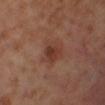Recorded during total-body skin imaging; not selected for excision or biopsy. The recorded lesion diameter is about 3.5 mm. Imaged with cross-polarized lighting. An algorithmic analysis of the crop reported a mean CIELAB color near L≈36 a*≈21 b*≈26 and a lesion–skin lightness drop of about 7. It also reported a border-irregularity rating of about 2.5/10 and radial color variation of about 1. And it measured a detector confidence of about 100 out of 100 that the crop contains a lesion. A lesion tile, about 15 mm wide, cut from a 3D total-body photograph. The lesion is located on the leg. A male subject, aged 68 to 72.